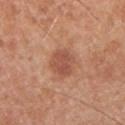<record>
  <biopsy_status>not biopsied; imaged during a skin examination</biopsy_status>
  <site>chest</site>
  <lighting>white-light</lighting>
  <patient>
    <sex>male</sex>
    <age_approx>30</age_approx>
  </patient>
  <lesion_size>
    <long_diameter_mm_approx>3.0</long_diameter_mm_approx>
  </lesion_size>
  <image>
    <source>total-body photography crop</source>
    <field_of_view_mm>15</field_of_view_mm>
  </image>
</record>